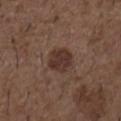Assessment:
Captured during whole-body skin photography for melanoma surveillance; the lesion was not biopsied.
Acquisition and patient details:
A close-up tile cropped from a whole-body skin photograph, about 15 mm across. The tile uses white-light illumination. The recorded lesion diameter is about 3.5 mm. The lesion is on the right forearm. A male subject, in their 50s.